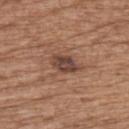Captured during whole-body skin photography for melanoma surveillance; the lesion was not biopsied.